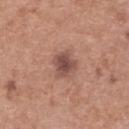Assessment:
This lesion was catalogued during total-body skin photography and was not selected for biopsy.
Context:
A female subject in their 60s. On the upper back. The tile uses white-light illumination. The lesion-visualizer software estimated an average lesion color of about L≈49 a*≈21 b*≈24 (CIELAB), a lesion–skin lightness drop of about 12, and a lesion-to-skin contrast of about 8.5 (normalized; higher = more distinct). A close-up tile cropped from a whole-body skin photograph, about 15 mm across. Longest diameter approximately 3 mm.